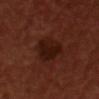No biopsy was performed on this lesion — it was imaged during a full skin examination and was not determined to be concerning. This is a cross-polarized tile. A 15 mm close-up extracted from a 3D total-body photography capture. A male subject, in their 50s. The lesion is on the chest. Longest diameter approximately 4 mm.